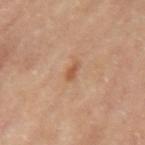Findings:
– follow-up: total-body-photography surveillance lesion; no biopsy
– body site: the left upper arm
– patient: female, approximately 65 years of age
– image: 15 mm crop, total-body photography